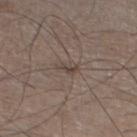The lesion was tiled from a total-body skin photograph and was not biopsied. A male subject approximately 60 years of age. The lesion is on the left lower leg. A close-up tile cropped from a whole-body skin photograph, about 15 mm across. The lesion-visualizer software estimated a mean CIELAB color near L≈43 a*≈12 b*≈20, about 7 CIELAB-L* units darker than the surrounding skin, and a normalized border contrast of about 6. And it measured border irregularity of about 5 on a 0–10 scale, internal color variation of about 0 on a 0–10 scale, and radial color variation of about 0. The analysis additionally found a classifier nevus-likeness of about 0/100 and a lesion-detection confidence of about 90/100. The tile uses white-light illumination.